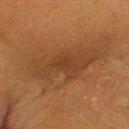The lesion is located on the head or neck. Approximately 8.5 mm at its widest. Automated image analysis of the tile measured a footprint of about 22 mm², an outline eccentricity of about 0.9 (0 = round, 1 = elongated), and a symmetry-axis asymmetry near 0.25. The analysis additionally found a peripheral color-asymmetry measure near 1. A region of skin cropped from a whole-body photographic capture, roughly 15 mm wide. The subject is a female roughly 55 years of age. Captured under cross-polarized illumination.